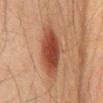<tbp_lesion>
<biopsy_status>not biopsied; imaged during a skin examination</biopsy_status>
<patient>
  <sex>male</sex>
  <age_approx>60</age_approx>
</patient>
<lesion_size>
  <long_diameter_mm_approx>5.5</long_diameter_mm_approx>
</lesion_size>
<site>chest</site>
<image>
  <source>total-body photography crop</source>
  <field_of_view_mm>15</field_of_view_mm>
</image>
<lighting>cross-polarized</lighting>
</tbp_lesion>